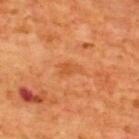Impression:
The lesion was photographed on a routine skin check and not biopsied; there is no pathology result.
Background:
The total-body-photography lesion software estimated an area of roughly 3 mm², an eccentricity of roughly 0.85, and two-axis asymmetry of about 0.35. The analysis additionally found an average lesion color of about L≈54 a*≈30 b*≈44 (CIELAB) and a lesion-to-skin contrast of about 5 (normalized; higher = more distinct). And it measured border irregularity of about 4 on a 0–10 scale, a color-variation rating of about 1/10, and peripheral color asymmetry of about 0.5. And it measured an automated nevus-likeness rating near 0 out of 100 and lesion-presence confidence of about 100/100. The subject is a male aged around 65. Longest diameter approximately 2.5 mm. The lesion is on the upper back. Cropped from a whole-body photographic skin survey; the tile spans about 15 mm. Imaged with cross-polarized lighting.